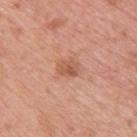biopsy status: no biopsy performed (imaged during a skin exam); lesion size: about 2.5 mm; illumination: white-light illumination; site: the upper back; TBP lesion metrics: an average lesion color of about L≈57 a*≈25 b*≈32 (CIELAB), a lesion–skin lightness drop of about 9, and a normalized lesion–skin contrast near 6; imaging modality: ~15 mm crop, total-body skin-cancer survey; patient: female, aged around 45.